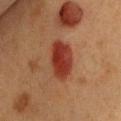Part of a total-body skin-imaging series; this lesion was reviewed on a skin check and was not flagged for biopsy. The lesion is on the chest. The patient is a female approximately 40 years of age. A close-up tile cropped from a whole-body skin photograph, about 15 mm across. Approximately 5.5 mm at its widest.